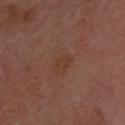Case summary:
- notes — no biopsy performed (imaged during a skin exam)
- body site — the head or neck
- subject — aged 63 to 67
- automated lesion analysis — a lesion area of about 3 mm², an eccentricity of roughly 0.85, and a shape-asymmetry score of about 0.25 (0 = symmetric); roughly 4 lightness units darker than nearby skin and a normalized border contrast of about 5; border irregularity of about 2.5 on a 0–10 scale and peripheral color asymmetry of about 0.5; an automated nevus-likeness rating near 0 out of 100 and a detector confidence of about 100 out of 100 that the crop contains a lesion
- imaging modality — 15 mm crop, total-body photography
- diameter — about 2.5 mm
- illumination — cross-polarized